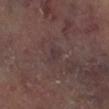Notes:
* workup: catalogued during a skin exam; not biopsied
* body site: the left lower leg
* lighting: cross-polarized illumination
* subject: male, aged around 65
* acquisition: total-body-photography crop, ~15 mm field of view